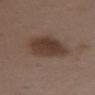Impression:
Part of a total-body skin-imaging series; this lesion was reviewed on a skin check and was not flagged for biopsy.
Context:
The tile uses white-light illumination. The lesion's longest dimension is about 6 mm. A close-up tile cropped from a whole-body skin photograph, about 15 mm across. A female patient, aged around 35. From the mid back.